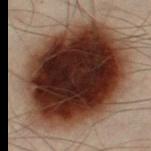Clinical impression: Imaged during a routine full-body skin examination; the lesion was not biopsied and no histopathology is available. Acquisition and patient details: Longest diameter approximately 12 mm. The lesion is on the left leg. This image is a 15 mm lesion crop taken from a total-body photograph. Imaged with cross-polarized lighting. The subject is a male aged 48 to 52.